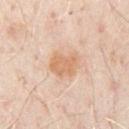| field | value |
|---|---|
| workup | imaged on a skin check; not biopsied |
| body site | the chest |
| illumination | cross-polarized |
| size | ≈3.5 mm |
| subject | male, aged 48 to 52 |
| image source | total-body-photography crop, ~15 mm field of view |